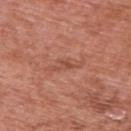{
  "biopsy_status": "not biopsied; imaged during a skin examination",
  "site": "upper back",
  "patient": {
    "sex": "male",
    "age_approx": 70
  },
  "lighting": "white-light",
  "image": {
    "source": "total-body photography crop",
    "field_of_view_mm": 15
  },
  "lesion_size": {
    "long_diameter_mm_approx": 4.0
  }
}